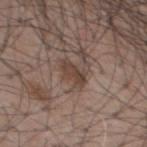| field | value |
|---|---|
| notes | no biopsy performed (imaged during a skin exam) |
| imaging modality | ~15 mm crop, total-body skin-cancer survey |
| subject | male, in their mid-50s |
| anatomic site | the chest |
| automated lesion analysis | an area of roughly 5 mm² and a shape-asymmetry score of about 0.25 (0 = symmetric); a border-irregularity index near 3/10 and internal color variation of about 2.5 on a 0–10 scale |
| diameter | about 3.5 mm |
| illumination | white-light illumination |